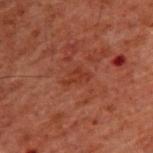Assessment: Captured during whole-body skin photography for melanoma surveillance; the lesion was not biopsied. Background: A male subject roughly 60 years of age. A 15 mm close-up extracted from a 3D total-body photography capture. About 3 mm across. On the upper back. Captured under cross-polarized illumination.